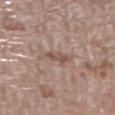Imaged during a routine full-body skin examination; the lesion was not biopsied and no histopathology is available. A male patient aged 68–72. From the right lower leg. A close-up tile cropped from a whole-body skin photograph, about 15 mm across. Automated image analysis of the tile measured an area of roughly 2.5 mm², a shape eccentricity near 0.9, and two-axis asymmetry of about 0.35. The analysis additionally found roughly 9 lightness units darker than nearby skin and a lesion-to-skin contrast of about 7 (normalized; higher = more distinct). And it measured border irregularity of about 4 on a 0–10 scale, a color-variation rating of about 0/10, and peripheral color asymmetry of about 0. The analysis additionally found a classifier nevus-likeness of about 0/100 and a lesion-detection confidence of about 100/100.